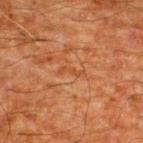Clinical summary:
A 15 mm close-up extracted from a 3D total-body photography capture. Imaged with cross-polarized lighting. On the upper back. Longest diameter approximately 3 mm. An algorithmic analysis of the crop reported roughly 4 lightness units darker than nearby skin and a normalized border contrast of about 4.5. It also reported a nevus-likeness score of about 0/100 and a detector confidence of about 55 out of 100 that the crop contains a lesion. A male subject, aged around 60.